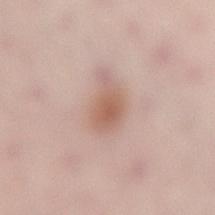Assessment:
No biopsy was performed on this lesion — it was imaged during a full skin examination and was not determined to be concerning.
Clinical summary:
The lesion is located on the lower back. Captured under white-light illumination. A female patient in their 30s. A lesion tile, about 15 mm wide, cut from a 3D total-body photograph.